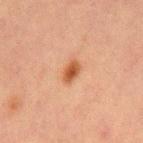Q: Was a biopsy performed?
A: total-body-photography surveillance lesion; no biopsy